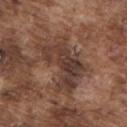Case summary:
- biopsy status · total-body-photography surveillance lesion; no biopsy
- lesion size · about 7 mm
- tile lighting · white-light illumination
- subject · male, aged around 75
- anatomic site · the upper back
- imaging modality · ~15 mm crop, total-body skin-cancer survey